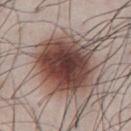Q: Is there a histopathology result?
A: total-body-photography surveillance lesion; no biopsy
Q: What lighting was used for the tile?
A: white-light illumination
Q: Patient demographics?
A: male, in their mid- to late 30s
Q: What kind of image is this?
A: 15 mm crop, total-body photography
Q: Lesion location?
A: the abdomen